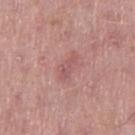biopsy status = catalogued during a skin exam; not biopsied | anatomic site = the left thigh | patient = female, about 60 years old | lesion size = about 3.5 mm | acquisition = 15 mm crop, total-body photography.